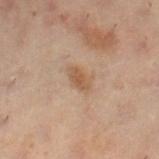Part of a total-body skin-imaging series; this lesion was reviewed on a skin check and was not flagged for biopsy. The lesion is located on the leg. A female subject, approximately 55 years of age. The tile uses cross-polarized illumination. A lesion tile, about 15 mm wide, cut from a 3D total-body photograph. Approximately 3 mm at its widest.